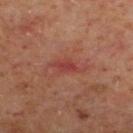Q: Was a biopsy performed?
A: imaged on a skin check; not biopsied
Q: What is the imaging modality?
A: 15 mm crop, total-body photography
Q: Automated lesion metrics?
A: a footprint of about 3 mm² and an outline eccentricity of about 0.8 (0 = round, 1 = elongated); a mean CIELAB color near L≈38 a*≈30 b*≈25 and a normalized lesion–skin contrast near 6; a border-irregularity index near 2.5/10, a color-variation rating of about 1/10, and peripheral color asymmetry of about 0; a classifier nevus-likeness of about 0/100 and lesion-presence confidence of about 100/100
Q: How large is the lesion?
A: ~2.5 mm (longest diameter)
Q: What is the anatomic site?
A: the left lower leg
Q: Patient demographics?
A: male, aged approximately 60
Q: What lighting was used for the tile?
A: cross-polarized illumination